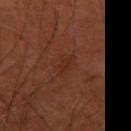<case>
  <biopsy_status>not biopsied; imaged during a skin examination</biopsy_status>
  <automated_metrics>
    <eccentricity>0.9</eccentricity>
    <shape_asymmetry>0.4</shape_asymmetry>
    <cielab_L>26</cielab_L>
    <cielab_a>23</cielab_a>
    <cielab_b>27</cielab_b>
    <vs_skin_contrast_norm>5.0</vs_skin_contrast_norm>
    <border_irregularity_0_10>4.5</border_irregularity_0_10>
    <color_variation_0_10>0.0</color_variation_0_10>
    <peripheral_color_asymmetry>0.0</peripheral_color_asymmetry>
  </automated_metrics>
  <lesion_size>
    <long_diameter_mm_approx>2.5</long_diameter_mm_approx>
  </lesion_size>
  <site>right thigh</site>
  <lighting>cross-polarized</lighting>
  <patient>
    <sex>male</sex>
    <age_approx>65</age_approx>
  </patient>
  <image>
    <source>total-body photography crop</source>
    <field_of_view_mm>15</field_of_view_mm>
  </image>
</case>